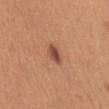<record>
  <biopsy_status>not biopsied; imaged during a skin examination</biopsy_status>
  <lighting>white-light</lighting>
  <patient>
    <sex>female</sex>
    <age_approx>30</age_approx>
  </patient>
  <site>mid back</site>
  <image>
    <source>total-body photography crop</source>
    <field_of_view_mm>15</field_of_view_mm>
  </image>
  <lesion_size>
    <long_diameter_mm_approx>2.5</long_diameter_mm_approx>
  </lesion_size>
</record>